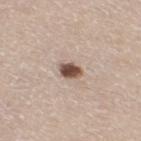{"biopsy_status": "not biopsied; imaged during a skin examination", "site": "right thigh", "lesion_size": {"long_diameter_mm_approx": 2.5}, "automated_metrics": {"area_mm2_approx": 4.5, "eccentricity": 0.65, "shape_asymmetry": 0.25, "border_irregularity_0_10": 2.5, "color_variation_0_10": 4.0, "nevus_likeness_0_100": 100, "lesion_detection_confidence_0_100": 100}, "image": {"source": "total-body photography crop", "field_of_view_mm": 15}, "patient": {"sex": "female", "age_approx": 45}, "lighting": "white-light"}